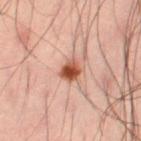{"biopsy_status": "not biopsied; imaged during a skin examination", "patient": {"sex": "male", "age_approx": 35}, "lighting": "cross-polarized", "image": {"source": "total-body photography crop", "field_of_view_mm": 15}, "site": "left thigh"}